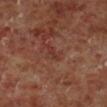Captured during whole-body skin photography for melanoma surveillance; the lesion was not biopsied.
An algorithmic analysis of the crop reported a footprint of about 2.5 mm² and an eccentricity of roughly 0.9.
This is a cross-polarized tile.
A close-up tile cropped from a whole-body skin photograph, about 15 mm across.
A male subject aged approximately 60.
The lesion is located on the right lower leg.
The recorded lesion diameter is about 2.5 mm.